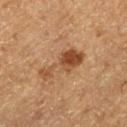biopsy status = catalogued during a skin exam; not biopsied | lesion size = ~5.5 mm (longest diameter) | image source = 15 mm crop, total-body photography | lighting = cross-polarized | patient = male, aged approximately 75 | body site = the leg | TBP lesion metrics = a footprint of about 10 mm² and a shape eccentricity near 0.9; a nevus-likeness score of about 45/100 and lesion-presence confidence of about 100/100.